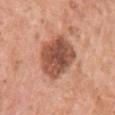{"biopsy_status": "not biopsied; imaged during a skin examination", "site": "left upper arm", "patient": {"sex": "female", "age_approx": 50}, "lesion_size": {"long_diameter_mm_approx": 6.0}, "image": {"source": "total-body photography crop", "field_of_view_mm": 15}}